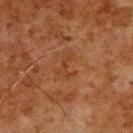Imaged during a routine full-body skin examination; the lesion was not biopsied and no histopathology is available.
A male patient, aged 78 to 82.
The lesion is on the upper back.
This image is a 15 mm lesion crop taken from a total-body photograph.